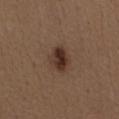Q: Was a biopsy performed?
A: total-body-photography surveillance lesion; no biopsy
Q: Patient demographics?
A: female, in their 40s
Q: Where on the body is the lesion?
A: the abdomen
Q: What is the imaging modality?
A: 15 mm crop, total-body photography